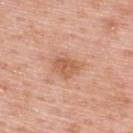biopsy status = total-body-photography surveillance lesion; no biopsy
location = the upper back
subject = male, aged 58 to 62
acquisition = total-body-photography crop, ~15 mm field of view